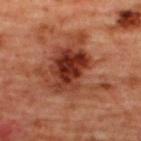Q: Was a biopsy performed?
A: total-body-photography surveillance lesion; no biopsy
Q: What is the anatomic site?
A: the upper back
Q: What are the patient's age and sex?
A: female, aged approximately 45
Q: How large is the lesion?
A: ≈7.5 mm
Q: What kind of image is this?
A: 15 mm crop, total-body photography